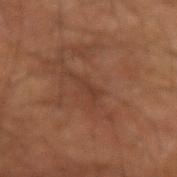workup = no biopsy performed (imaged during a skin exam) | diameter = ~3 mm (longest diameter) | acquisition = ~15 mm crop, total-body skin-cancer survey | patient = male, aged around 70 | anatomic site = the left forearm.